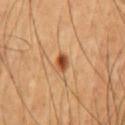notes = catalogued during a skin exam; not biopsied | imaging modality = 15 mm crop, total-body photography | lesion size = about 2 mm | illumination = cross-polarized | body site = the chest | patient = male, aged approximately 55.